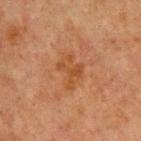Clinical impression:
Part of a total-body skin-imaging series; this lesion was reviewed on a skin check and was not flagged for biopsy.
Acquisition and patient details:
The lesion is on the chest. A male patient in their mid-60s. A region of skin cropped from a whole-body photographic capture, roughly 15 mm wide. Automated image analysis of the tile measured a footprint of about 8.5 mm², a shape eccentricity near 0.75, and a shape-asymmetry score of about 0.35 (0 = symmetric). It also reported a lesion–skin lightness drop of about 6 and a lesion-to-skin contrast of about 6 (normalized; higher = more distinct). The analysis additionally found a nevus-likeness score of about 0/100 and a detector confidence of about 100 out of 100 that the crop contains a lesion. Measured at roughly 4 mm in maximum diameter. Imaged with cross-polarized lighting.